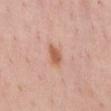Context:
The lesion-visualizer software estimated an area of roughly 4 mm², an eccentricity of roughly 0.75, and two-axis asymmetry of about 0.3. On the chest. A male subject, in their 60s. About 2.5 mm across. A 15 mm crop from a total-body photograph taken for skin-cancer surveillance. Imaged with white-light lighting.